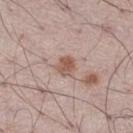Findings:
* workup: imaged on a skin check; not biopsied
* anatomic site: the right thigh
* image source: ~15 mm crop, total-body skin-cancer survey
* lighting: white-light
* automated metrics: a lesion color around L≈56 a*≈18 b*≈26 in CIELAB, about 11 CIELAB-L* units darker than the surrounding skin, and a normalized border contrast of about 7.5
* patient: male, aged 48 to 52